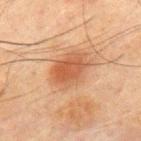Clinical impression:
Imaged during a routine full-body skin examination; the lesion was not biopsied and no histopathology is available.
Background:
The lesion is on the front of the torso. Automated image analysis of the tile measured a shape eccentricity near 0.75 and a shape-asymmetry score of about 0.15 (0 = symmetric). It also reported border irregularity of about 1.5 on a 0–10 scale, a within-lesion color-variation index near 4/10, and peripheral color asymmetry of about 1. The software also gave a nevus-likeness score of about 95/100. Cropped from a whole-body photographic skin survey; the tile spans about 15 mm. The subject is a male approximately 70 years of age. Imaged with cross-polarized lighting.